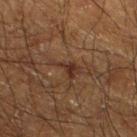Recorded during total-body skin imaging; not selected for excision or biopsy. Automated image analysis of the tile measured a footprint of about 4 mm² and an outline eccentricity of about 0.85 (0 = round, 1 = elongated). The software also gave a mean CIELAB color near L≈26 a*≈16 b*≈22, roughly 6 lightness units darker than nearby skin, and a normalized border contrast of about 7.5. The analysis additionally found border irregularity of about 7.5 on a 0–10 scale and a color-variation rating of about 2/10. Cropped from a whole-body photographic skin survey; the tile spans about 15 mm. From the leg. The subject is a male in their mid- to late 60s.